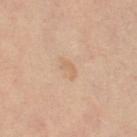patient = female, aged around 50; illumination = cross-polarized illumination; acquisition = 15 mm crop, total-body photography; diameter = about 2.5 mm; body site = the left thigh.